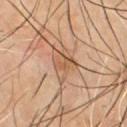The lesion was photographed on a routine skin check and not biopsied; there is no pathology result. A male patient, aged 53 to 57. The lesion's longest dimension is about 4.5 mm. A 15 mm close-up tile from a total-body photography series done for melanoma screening. Located on the chest.